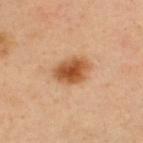<tbp_lesion>
  <image>
    <source>total-body photography crop</source>
    <field_of_view_mm>15</field_of_view_mm>
  </image>
  <automated_metrics>
    <nevus_likeness_0_100>100</nevus_likeness_0_100>
    <lesion_detection_confidence_0_100>100</lesion_detection_confidence_0_100>
  </automated_metrics>
  <site>upper back</site>
  <lesion_size>
    <long_diameter_mm_approx>4.0</long_diameter_mm_approx>
  </lesion_size>
  <patient>
    <sex>male</sex>
    <age_approx>35</age_approx>
  </patient>
  <lighting>cross-polarized</lighting>
</tbp_lesion>